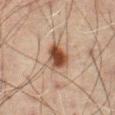Assessment: Captured during whole-body skin photography for melanoma surveillance; the lesion was not biopsied.